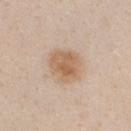Part of a total-body skin-imaging series; this lesion was reviewed on a skin check and was not flagged for biopsy. Automated image analysis of the tile measured an automated nevus-likeness rating near 80 out of 100 and lesion-presence confidence of about 100/100. Approximately 4 mm at its widest. Cropped from a total-body skin-imaging series; the visible field is about 15 mm. The lesion is located on the chest. The patient is a female aged approximately 20. This is a white-light tile.